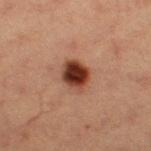subject — female, aged 38–42
image-analysis metrics — an outline eccentricity of about 0.6 (0 = round, 1 = elongated) and a symmetry-axis asymmetry near 0.15; a border-irregularity rating of about 1.5/10, internal color variation of about 6.5 on a 0–10 scale, and a peripheral color-asymmetry measure near 2; a detector confidence of about 100 out of 100 that the crop contains a lesion
image — ~15 mm crop, total-body skin-cancer survey
illumination — cross-polarized illumination
lesion diameter — ~3.5 mm (longest diameter)
location — the leg